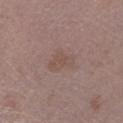– biopsy status — no biopsy performed (imaged during a skin exam)
– anatomic site — the left lower leg
– acquisition — ~15 mm crop, total-body skin-cancer survey
– size — ≈3.5 mm
– subject — male, about 45 years old